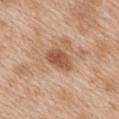{"biopsy_status": "not biopsied; imaged during a skin examination", "image": {"source": "total-body photography crop", "field_of_view_mm": 15}, "patient": {"sex": "female", "age_approx": 40}, "site": "mid back"}